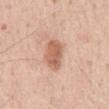<record>
<biopsy_status>not biopsied; imaged during a skin examination</biopsy_status>
<patient>
  <sex>male</sex>
  <age_approx>55</age_approx>
</patient>
<image>
  <source>total-body photography crop</source>
  <field_of_view_mm>15</field_of_view_mm>
</image>
<lesion_size>
  <long_diameter_mm_approx>4.0</long_diameter_mm_approx>
</lesion_size>
<site>front of the torso</site>
</record>